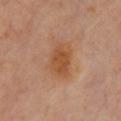Q: Was a biopsy performed?
A: total-body-photography surveillance lesion; no biopsy
Q: How large is the lesion?
A: ≈4.5 mm
Q: Where on the body is the lesion?
A: the chest
Q: What kind of image is this?
A: ~15 mm crop, total-body skin-cancer survey
Q: Patient demographics?
A: female, aged 58 to 62